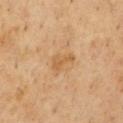Context:
A male patient aged around 70. An algorithmic analysis of the crop reported a lesion–skin lightness drop of about 7 and a normalized border contrast of about 6. And it measured a border-irregularity rating of about 4.5/10, a color-variation rating of about 1.5/10, and a peripheral color-asymmetry measure near 0.5. And it measured an automated nevus-likeness rating near 0 out of 100 and a detector confidence of about 100 out of 100 that the crop contains a lesion. A roughly 15 mm field-of-view crop from a total-body skin photograph. About 3 mm across. On the front of the torso. Captured under cross-polarized illumination.